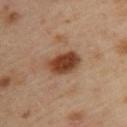Part of a total-body skin-imaging series; this lesion was reviewed on a skin check and was not flagged for biopsy.
The recorded lesion diameter is about 4.5 mm.
Imaged with cross-polarized lighting.
Located on the upper back.
The patient is a female about 40 years old.
A 15 mm close-up tile from a total-body photography series done for melanoma screening.